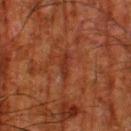Part of a total-body skin-imaging series; this lesion was reviewed on a skin check and was not flagged for biopsy.
Captured under cross-polarized illumination.
The lesion is on the left thigh.
A male subject, aged approximately 80.
Cropped from a total-body skin-imaging series; the visible field is about 15 mm.